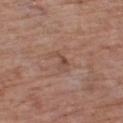This is a white-light tile. On the right thigh. A male patient, in their mid- to late 70s. This image is a 15 mm lesion crop taken from a total-body photograph.